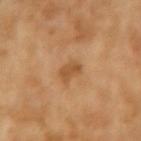biopsy status: total-body-photography surveillance lesion; no biopsy
subject: female, aged 68–72
anatomic site: the left forearm
tile lighting: cross-polarized illumination
TBP lesion metrics: a footprint of about 3.5 mm², a shape eccentricity near 0.9, and a symmetry-axis asymmetry near 0.35; a lesion color around L≈53 a*≈22 b*≈40 in CIELAB, a lesion–skin lightness drop of about 9, and a normalized border contrast of about 7; an automated nevus-likeness rating near 0 out of 100 and lesion-presence confidence of about 100/100
acquisition: ~15 mm tile from a whole-body skin photo
lesion size: ~3 mm (longest diameter)